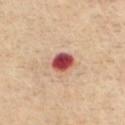Captured during whole-body skin photography for melanoma surveillance; the lesion was not biopsied.
A male patient approximately 70 years of age.
A 15 mm close-up tile from a total-body photography series done for melanoma screening.
The tile uses cross-polarized illumination.
On the chest.